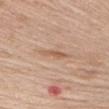workup: no biopsy performed (imaged during a skin exam) | imaging modality: ~15 mm tile from a whole-body skin photo | lighting: white-light | patient: male, aged approximately 75 | anatomic site: the right upper arm | TBP lesion metrics: an average lesion color of about L≈58 a*≈20 b*≈32 (CIELAB), roughly 9 lightness units darker than nearby skin, and a lesion-to-skin contrast of about 6 (normalized; higher = more distinct); a border-irregularity rating of about 2.5/10, a color-variation rating of about 0.5/10, and peripheral color asymmetry of about 0 | size: ≈3 mm.